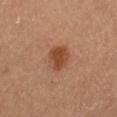<lesion>
<biopsy_status>not biopsied; imaged during a skin examination</biopsy_status>
<site>left thigh</site>
<lighting>cross-polarized</lighting>
<automated_metrics>
  <shape_asymmetry>0.25</shape_asymmetry>
  <vs_skin_darker_L>11.0</vs_skin_darker_L>
  <vs_skin_contrast_norm>8.5</vs_skin_contrast_norm>
  <border_irregularity_0_10>2.0</border_irregularity_0_10>
  <color_variation_0_10>2.0</color_variation_0_10>
  <peripheral_color_asymmetry>0.5</peripheral_color_asymmetry>
  <nevus_likeness_0_100>100</nevus_likeness_0_100>
</automated_metrics>
<patient>
  <sex>female</sex>
  <age_approx>60</age_approx>
</patient>
<lesion_size>
  <long_diameter_mm_approx>3.0</long_diameter_mm_approx>
</lesion_size>
<image>
  <source>total-body photography crop</source>
  <field_of_view_mm>15</field_of_view_mm>
</image>
</lesion>